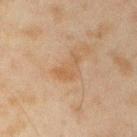{"biopsy_status": "not biopsied; imaged during a skin examination", "image": {"source": "total-body photography crop", "field_of_view_mm": 15}, "lesion_size": {"long_diameter_mm_approx": 3.5}, "patient": {"sex": "male", "age_approx": 45}, "site": "right upper arm", "automated_metrics": {"area_mm2_approx": 4.5, "eccentricity": 0.85, "shape_asymmetry": 0.65}}